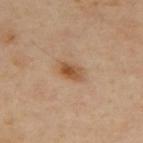Recorded during total-body skin imaging; not selected for excision or biopsy. This image is a 15 mm lesion crop taken from a total-body photograph. About 3 mm across. Automated tile analysis of the lesion measured roughly 10 lightness units darker than nearby skin and a lesion-to-skin contrast of about 8 (normalized; higher = more distinct). The software also gave a classifier nevus-likeness of about 90/100 and a detector confidence of about 100 out of 100 that the crop contains a lesion. The lesion is located on the upper back. A male patient in their mid- to late 60s.